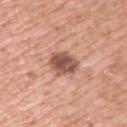On the upper back. A lesion tile, about 15 mm wide, cut from a 3D total-body photograph. The subject is a female in their 40s.